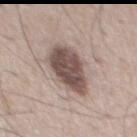The total-body-photography lesion software estimated a border-irregularity index near 2/10 and a color-variation rating of about 4.5/10. Imaged with white-light lighting. This image is a 15 mm lesion crop taken from a total-body photograph. The patient is a male aged 53–57. Measured at roughly 5.5 mm in maximum diameter.